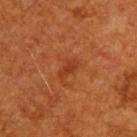Case summary:
• notes: total-body-photography surveillance lesion; no biopsy
• lesion size: about 3 mm
• body site: the upper back
• patient: male, roughly 60 years of age
• image source: 15 mm crop, total-body photography
• illumination: cross-polarized